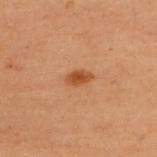Notes:
• biopsy status — catalogued during a skin exam; not biopsied
• TBP lesion metrics — an area of roughly 4 mm² and a shape eccentricity near 0.8; a border-irregularity rating of about 2/10 and peripheral color asymmetry of about 0.5; a nevus-likeness score of about 100/100 and lesion-presence confidence of about 100/100
• imaging modality — ~15 mm crop, total-body skin-cancer survey
• location — the upper back
• lesion size — ≈2.5 mm
• patient — female, aged 48 to 52
• illumination — cross-polarized illumination